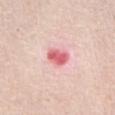Assessment: Imaged during a routine full-body skin examination; the lesion was not biopsied and no histopathology is available. Context: A female subject, approximately 45 years of age. The lesion's longest dimension is about 3 mm. A lesion tile, about 15 mm wide, cut from a 3D total-body photograph. This is a white-light tile. An algorithmic analysis of the crop reported an area of roughly 5 mm², an outline eccentricity of about 0.75 (0 = round, 1 = elongated), and a symmetry-axis asymmetry near 0.2. The analysis additionally found a mean CIELAB color near L≈64 a*≈37 b*≈23, about 15 CIELAB-L* units darker than the surrounding skin, and a normalized border contrast of about 9. The software also gave radial color variation of about 1. And it measured a classifier nevus-likeness of about 0/100. Located on the abdomen.